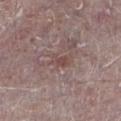The lesion was photographed on a routine skin check and not biopsied; there is no pathology result. A 15 mm crop from a total-body photograph taken for skin-cancer surveillance. This is a white-light tile. Measured at roughly 2.5 mm in maximum diameter. A male subject aged around 75. Located on the left lower leg. The total-body-photography lesion software estimated a footprint of about 3 mm², a shape eccentricity near 0.85, and a symmetry-axis asymmetry near 0.3. And it measured internal color variation of about 1 on a 0–10 scale. The software also gave an automated nevus-likeness rating near 0 out of 100 and lesion-presence confidence of about 95/100.